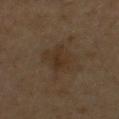Q: Is there a histopathology result?
A: no biopsy performed (imaged during a skin exam)
Q: What is the anatomic site?
A: the upper back
Q: What kind of image is this?
A: 15 mm crop, total-body photography
Q: What are the patient's age and sex?
A: male, approximately 60 years of age
Q: What did automated image analysis measure?
A: a lesion color around L≈29 a*≈12 b*≈24 in CIELAB and a lesion–skin lightness drop of about 5; a nevus-likeness score of about 5/100 and a detector confidence of about 100 out of 100 that the crop contains a lesion